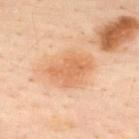Captured during whole-body skin photography for melanoma surveillance; the lesion was not biopsied.
The patient is a male aged 48–52.
Cropped from a total-body skin-imaging series; the visible field is about 15 mm.
Located on the upper back.